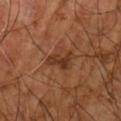biopsy_status: not biopsied; imaged during a skin examination
automated_metrics:
  eccentricity: 0.8
  shape_asymmetry: 0.4
  cielab_L: 33
  cielab_a: 22
  cielab_b: 31
  vs_skin_darker_L: 8.0
  vs_skin_contrast_norm: 8.0
  color_variation_0_10: 2.5
  peripheral_color_asymmetry: 1.0
patient:
  sex: male
  age_approx: 60
lighting: cross-polarized
image:
  source: total-body photography crop
  field_of_view_mm: 15
site: right arm
lesion_size:
  long_diameter_mm_approx: 3.5